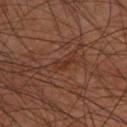An algorithmic analysis of the crop reported an average lesion color of about L≈30 a*≈21 b*≈27 (CIELAB), a lesion–skin lightness drop of about 5, and a lesion-to-skin contrast of about 6 (normalized; higher = more distinct). Longest diameter approximately 2.5 mm. Cropped from a whole-body photographic skin survey; the tile spans about 15 mm. Located on the upper back. A male patient about 45 years old. Imaged with cross-polarized lighting.